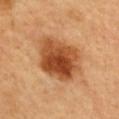biopsy status: total-body-photography surveillance lesion; no biopsy
imaging modality: 15 mm crop, total-body photography
subject: female, aged around 60
anatomic site: the upper back